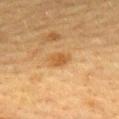The lesion was tiled from a total-body skin photograph and was not biopsied. The lesion is on the upper back. A 15 mm crop from a total-body photograph taken for skin-cancer surveillance. Approximately 2.5 mm at its widest. A female subject roughly 55 years of age. The lesion-visualizer software estimated a border-irregularity rating of about 2/10 and a peripheral color-asymmetry measure near 1.